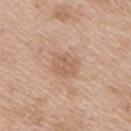Clinical impression:
Captured during whole-body skin photography for melanoma surveillance; the lesion was not biopsied.
Background:
The recorded lesion diameter is about 3.5 mm. The patient is a female aged around 40. From the right upper arm. A lesion tile, about 15 mm wide, cut from a 3D total-body photograph. The tile uses white-light illumination.